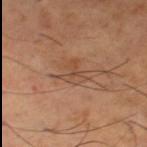Findings:
– image — ~15 mm crop, total-body skin-cancer survey
– lesion diameter — ~2.5 mm (longest diameter)
– lighting — cross-polarized illumination
– anatomic site — the right thigh
– subject — male, roughly 65 years of age